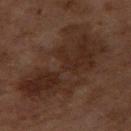This lesion was catalogued during total-body skin photography and was not selected for biopsy.